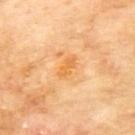Impression:
Imaged during a routine full-body skin examination; the lesion was not biopsied and no histopathology is available.
Context:
Captured under cross-polarized illumination. This image is a 15 mm lesion crop taken from a total-body photograph. A male patient aged around 70. Located on the mid back. The total-body-photography lesion software estimated a mean CIELAB color near L≈67 a*≈25 b*≈49, a lesion–skin lightness drop of about 7, and a normalized border contrast of about 6.5. Approximately 3 mm at its widest.